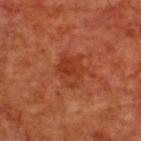follow-up = catalogued during a skin exam; not biopsied | patient = male, approximately 70 years of age | lighting = cross-polarized illumination | acquisition = 15 mm crop, total-body photography | anatomic site = the back.